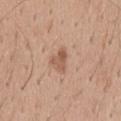Assessment: Captured during whole-body skin photography for melanoma surveillance; the lesion was not biopsied. Context: Cropped from a whole-body photographic skin survey; the tile spans about 15 mm. About 3.5 mm across. The subject is a male aged 38 to 42. The lesion is located on the mid back. Captured under white-light illumination.